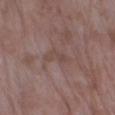workup: catalogued during a skin exam; not biopsied | size: about 4 mm | illumination: white-light illumination | automated metrics: an area of roughly 5.5 mm² and a symmetry-axis asymmetry near 0.45; border irregularity of about 5 on a 0–10 scale, internal color variation of about 2 on a 0–10 scale, and peripheral color asymmetry of about 0.5 | image source: total-body-photography crop, ~15 mm field of view | anatomic site: the left lower leg | patient: female, approximately 70 years of age.